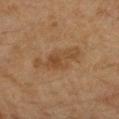lighting=cross-polarized illumination
automated metrics=a lesion area of about 12 mm², an eccentricity of roughly 0.95, and two-axis asymmetry of about 0.3; a lesion color around L≈43 a*≈17 b*≈32 in CIELAB; a classifier nevus-likeness of about 5/100 and a detector confidence of about 100 out of 100 that the crop contains a lesion
subject=female, aged around 60
anatomic site=the arm
acquisition=~15 mm tile from a whole-body skin photo